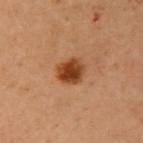Impression:
This lesion was catalogued during total-body skin photography and was not selected for biopsy.
Acquisition and patient details:
A female subject, aged 58 to 62. From the chest. Longest diameter approximately 3 mm. The tile uses cross-polarized illumination. This image is a 15 mm lesion crop taken from a total-body photograph.